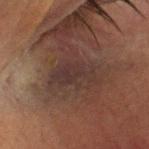• workup · imaged on a skin check; not biopsied
• site · the head or neck
• diameter · about 5.5 mm
• image source · total-body-photography crop, ~15 mm field of view
• patient · male, approximately 70 years of age
• automated metrics · a lesion color around L≈26 a*≈14 b*≈17 in CIELAB, about 5 CIELAB-L* units darker than the surrounding skin, and a normalized border contrast of about 6.5; a nevus-likeness score of about 0/100 and a detector confidence of about 80 out of 100 that the crop contains a lesion